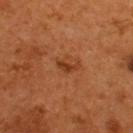Findings:
– biopsy status: no biopsy performed (imaged during a skin exam)
– body site: the upper back
– lighting: cross-polarized illumination
– imaging modality: ~15 mm crop, total-body skin-cancer survey
– diameter: about 2.5 mm
– patient: male, in their mid- to late 50s
– automated metrics: a border-irregularity index near 4/10, internal color variation of about 0 on a 0–10 scale, and a peripheral color-asymmetry measure near 0